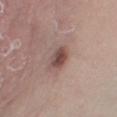Case summary:
* follow-up: imaged on a skin check; not biopsied